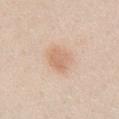<tbp_lesion>
  <biopsy_status>not biopsied; imaged during a skin examination</biopsy_status>
  <site>chest</site>
  <lesion_size>
    <long_diameter_mm_approx>3.5</long_diameter_mm_approx>
  </lesion_size>
  <lighting>white-light</lighting>
  <image>
    <source>total-body photography crop</source>
    <field_of_view_mm>15</field_of_view_mm>
  </image>
  <patient>
    <sex>female</sex>
    <age_approx>45</age_approx>
  </patient>
</tbp_lesion>